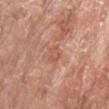The lesion was tiled from a total-body skin photograph and was not biopsied. From the head or neck. Imaged with white-light lighting. Cropped from a total-body skin-imaging series; the visible field is about 15 mm. The patient is a male about 65 years old.